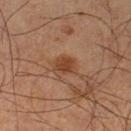Background:
Approximately 2.5 mm at its widest. A male patient, in their mid-60s. A lesion tile, about 15 mm wide, cut from a 3D total-body photograph. Located on the left lower leg. This is a cross-polarized tile.